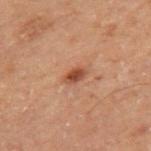This lesion was catalogued during total-body skin photography and was not selected for biopsy.
The subject is a male roughly 70 years of age.
The lesion-visualizer software estimated a lesion color around L≈37 a*≈21 b*≈26 in CIELAB, about 9 CIELAB-L* units darker than the surrounding skin, and a normalized border contrast of about 8. The analysis additionally found lesion-presence confidence of about 100/100.
A region of skin cropped from a whole-body photographic capture, roughly 15 mm wide.
The tile uses cross-polarized illumination.
The lesion is on the right thigh.
Longest diameter approximately 2.5 mm.